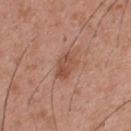Image and clinical context:
This is a white-light tile. Automated tile analysis of the lesion measured an area of roughly 5.5 mm², an eccentricity of roughly 0.75, and a symmetry-axis asymmetry near 0.2. And it measured a mean CIELAB color near L≈50 a*≈23 b*≈30 and a normalized lesion–skin contrast near 6.5. The lesion is on the upper back. A male subject, in their 30s. A close-up tile cropped from a whole-body skin photograph, about 15 mm across. About 3 mm across.